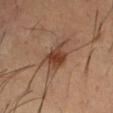Impression: The lesion was tiled from a total-body skin photograph and was not biopsied. Background: A male subject roughly 40 years of age. A lesion tile, about 15 mm wide, cut from a 3D total-body photograph. Imaged with cross-polarized lighting. The recorded lesion diameter is about 4 mm. On the left forearm.